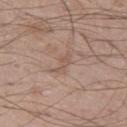Assessment: The lesion was photographed on a routine skin check and not biopsied; there is no pathology result. Acquisition and patient details: Cropped from a total-body skin-imaging series; the visible field is about 15 mm. From the left thigh. The subject is a male about 50 years old.